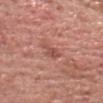No biopsy was performed on this lesion — it was imaged during a full skin examination and was not determined to be concerning. The patient is a male aged 68 to 72. The recorded lesion diameter is about 3 mm. A 15 mm crop from a total-body photograph taken for skin-cancer surveillance. An algorithmic analysis of the crop reported an area of roughly 3.5 mm², an outline eccentricity of about 0.8 (0 = round, 1 = elongated), and a shape-asymmetry score of about 0.35 (0 = symmetric). And it measured a lesion color around L≈51 a*≈26 b*≈27 in CIELAB, about 9 CIELAB-L* units darker than the surrounding skin, and a normalized border contrast of about 6.5. It also reported a border-irregularity rating of about 4/10 and radial color variation of about 0.5. This is a white-light tile. The lesion is located on the head or neck.